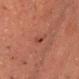Q: Is there a histopathology result?
A: no biopsy performed (imaged during a skin exam)
Q: What is the anatomic site?
A: the front of the torso
Q: What is the imaging modality?
A: 15 mm crop, total-body photography
Q: Automated lesion metrics?
A: an area of roughly 2 mm², a shape eccentricity near 0.95, and a shape-asymmetry score of about 0.55 (0 = symmetric); about 7 CIELAB-L* units darker than the surrounding skin and a normalized border contrast of about 6; a border-irregularity index near 5/10, a within-lesion color-variation index near 0/10, and peripheral color asymmetry of about 0
Q: How was the tile lit?
A: cross-polarized illumination
Q: Who is the patient?
A: male, in their mid-60s